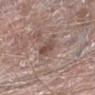Imaged during a routine full-body skin examination; the lesion was not biopsied and no histopathology is available.
The patient is a male in their mid- to late 60s.
An algorithmic analysis of the crop reported a footprint of about 5.5 mm² and a shape eccentricity near 0.7. And it measured a border-irregularity index near 2.5/10, a within-lesion color-variation index near 3/10, and radial color variation of about 1. It also reported a nevus-likeness score of about 0/100 and a lesion-detection confidence of about 95/100.
Longest diameter approximately 3 mm.
The lesion is on the left lower leg.
Imaged with white-light lighting.
Cropped from a total-body skin-imaging series; the visible field is about 15 mm.